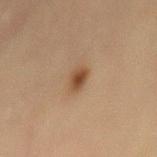{
  "biopsy_status": "not biopsied; imaged during a skin examination",
  "site": "mid back",
  "lesion_size": {
    "long_diameter_mm_approx": 2.5
  },
  "patient": {
    "sex": "female",
    "age_approx": 65
  },
  "lighting": "cross-polarized",
  "automated_metrics": {
    "area_mm2_approx": 3.5,
    "eccentricity": 0.8,
    "shape_asymmetry": 0.2,
    "cielab_L": 40,
    "cielab_a": 17,
    "cielab_b": 29,
    "vs_skin_darker_L": 10.0,
    "nevus_likeness_0_100": 95,
    "lesion_detection_confidence_0_100": 100
  },
  "image": {
    "source": "total-body photography crop",
    "field_of_view_mm": 15
  }
}